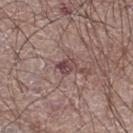Recorded during total-body skin imaging; not selected for excision or biopsy.
On the right thigh.
The lesion's longest dimension is about 2.5 mm.
The subject is a male in their mid-60s.
Cropped from a total-body skin-imaging series; the visible field is about 15 mm.
This is a white-light tile.
Automated image analysis of the tile measured a footprint of about 3.5 mm², an outline eccentricity of about 0.7 (0 = round, 1 = elongated), and a symmetry-axis asymmetry near 0.2. And it measured about 11 CIELAB-L* units darker than the surrounding skin and a lesion-to-skin contrast of about 8.5 (normalized; higher = more distinct). The software also gave a border-irregularity rating of about 2/10 and radial color variation of about 1.5. The software also gave a nevus-likeness score of about 0/100.